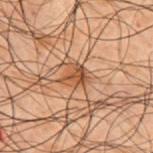biopsy status — total-body-photography surveillance lesion; no biopsy | image source — ~15 mm crop, total-body skin-cancer survey | size — about 2.5 mm | lighting — cross-polarized | anatomic site — the upper back | patient — male, aged 48 to 52.